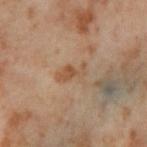Clinical impression: Recorded during total-body skin imaging; not selected for excision or biopsy. Acquisition and patient details: The lesion is located on the left thigh. A female patient, aged around 55. Automated tile analysis of the lesion measured a footprint of about 6 mm², a shape eccentricity near 0.8, and a shape-asymmetry score of about 0.35 (0 = symmetric). And it measured a mean CIELAB color near L≈54 a*≈18 b*≈33. The analysis additionally found a classifier nevus-likeness of about 0/100 and lesion-presence confidence of about 100/100. Cropped from a total-body skin-imaging series; the visible field is about 15 mm. Captured under cross-polarized illumination. The lesion's longest dimension is about 3.5 mm.